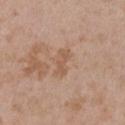No biopsy was performed on this lesion — it was imaged during a full skin examination and was not determined to be concerning.